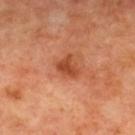Impression: Part of a total-body skin-imaging series; this lesion was reviewed on a skin check and was not flagged for biopsy. Context: The recorded lesion diameter is about 2.5 mm. The lesion is on the back. A roughly 15 mm field-of-view crop from a total-body skin photograph. A subject in their mid- to late 60s. This is a cross-polarized tile.